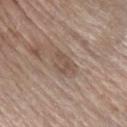Imaged during a routine full-body skin examination; the lesion was not biopsied and no histopathology is available.
Captured under white-light illumination.
A close-up tile cropped from a whole-body skin photograph, about 15 mm across.
From the right forearm.
The patient is a male aged 68 to 72.
Measured at roughly 3 mm in maximum diameter.